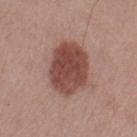{
  "biopsy_status": "not biopsied; imaged during a skin examination",
  "automated_metrics": {
    "cielab_L": 46,
    "cielab_a": 23,
    "cielab_b": 24,
    "vs_skin_darker_L": 14.0,
    "vs_skin_contrast_norm": 10.0,
    "nevus_likeness_0_100": 95
  },
  "image": {
    "source": "total-body photography crop",
    "field_of_view_mm": 15
  },
  "site": "left thigh",
  "lighting": "white-light",
  "patient": {
    "sex": "male",
    "age_approx": 70
  },
  "lesion_size": {
    "long_diameter_mm_approx": 6.0
  }
}